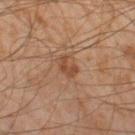Assessment:
Imaged during a routine full-body skin examination; the lesion was not biopsied and no histopathology is available.
Image and clinical context:
A male patient aged 43–47. The recorded lesion diameter is about 2.5 mm. Located on the left thigh. Cropped from a total-body skin-imaging series; the visible field is about 15 mm. The lesion-visualizer software estimated an average lesion color of about L≈37 a*≈18 b*≈27 (CIELAB), about 7 CIELAB-L* units darker than the surrounding skin, and a lesion-to-skin contrast of about 6.5 (normalized; higher = more distinct). It also reported a color-variation rating of about 2/10 and radial color variation of about 0.5. The analysis additionally found a classifier nevus-likeness of about 10/100 and a lesion-detection confidence of about 100/100. Imaged with cross-polarized lighting.